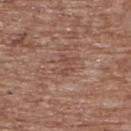  site: upper back
  lesion_size:
    long_diameter_mm_approx: 2.5
  patient:
    sex: female
    age_approx: 75
  image:
    source: total-body photography crop
    field_of_view_mm: 15